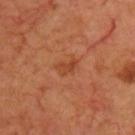Notes:
• subject — male, in their 70s
• image — ~15 mm tile from a whole-body skin photo
• illumination — cross-polarized
• site — the chest